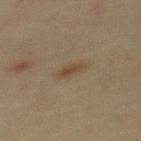The lesion was tiled from a total-body skin photograph and was not biopsied. The lesion is located on the mid back. A 15 mm close-up tile from a total-body photography series done for melanoma screening. A female patient, roughly 40 years of age.